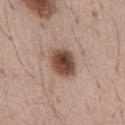follow-up — no biopsy performed (imaged during a skin exam); lesion size — about 4 mm; image-analysis metrics — a mean CIELAB color near L≈47 a*≈19 b*≈25, about 16 CIELAB-L* units darker than the surrounding skin, and a normalized lesion–skin contrast near 11.5; illumination — white-light illumination; image source — ~15 mm tile from a whole-body skin photo; patient — male, aged 53–57; body site — the abdomen.